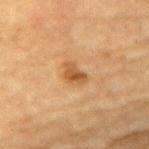Assessment: Captured during whole-body skin photography for melanoma surveillance; the lesion was not biopsied. Acquisition and patient details: The lesion is located on the mid back. A 15 mm close-up extracted from a 3D total-body photography capture. A male subject, in their mid- to late 80s or older.